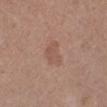| field | value |
|---|---|
| notes | catalogued during a skin exam; not biopsied |
| anatomic site | the left lower leg |
| tile lighting | white-light |
| image | 15 mm crop, total-body photography |
| patient | female, aged 53–57 |
| lesion size | ≈3.5 mm |